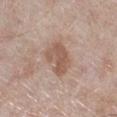| field | value |
|---|---|
| lighting | white-light illumination |
| acquisition | ~15 mm tile from a whole-body skin photo |
| patient | male, aged 68–72 |
| site | the leg |
| automated lesion analysis | an average lesion color of about L≈55 a*≈18 b*≈26 (CIELAB) and a lesion–skin lightness drop of about 10; an automated nevus-likeness rating near 25 out of 100 and a detector confidence of about 100 out of 100 that the crop contains a lesion |